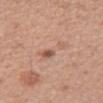biopsy status = total-body-photography surveillance lesion; no biopsy | lesion size = ≈5 mm | patient = female, approximately 35 years of age | anatomic site = the right forearm | acquisition = ~15 mm tile from a whole-body skin photo.